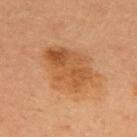follow-up: catalogued during a skin exam; not biopsied
image-analysis metrics: an area of roughly 21 mm², an outline eccentricity of about 0.7 (0 = round, 1 = elongated), and a symmetry-axis asymmetry near 0.25
illumination: cross-polarized
body site: the upper back
subject: female, about 35 years old
lesion diameter: about 6 mm
image source: total-body-photography crop, ~15 mm field of view